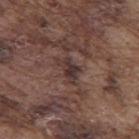No biopsy was performed on this lesion — it was imaged during a full skin examination and was not determined to be concerning.
A male patient, in their mid-70s.
About 3.5 mm across.
Cropped from a total-body skin-imaging series; the visible field is about 15 mm.
The lesion is on the back.
The tile uses white-light illumination.